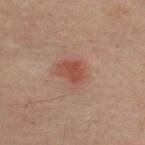Findings:
• notes: no biopsy performed (imaged during a skin exam)
• tile lighting: cross-polarized illumination
• automated lesion analysis: an area of roughly 8.5 mm², an outline eccentricity of about 0.6 (0 = round, 1 = elongated), and a shape-asymmetry score of about 0.25 (0 = symmetric); a border-irregularity rating of about 2.5/10, a color-variation rating of about 3/10, and radial color variation of about 1; a lesion-detection confidence of about 100/100
• patient: male, roughly 50 years of age
• lesion diameter: ≈3.5 mm
• body site: the upper back
• acquisition: 15 mm crop, total-body photography